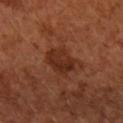Clinical impression:
This lesion was catalogued during total-body skin photography and was not selected for biopsy.
Acquisition and patient details:
This image is a 15 mm lesion crop taken from a total-body photograph. The lesion's longest dimension is about 4 mm. The lesion-visualizer software estimated an automated nevus-likeness rating near 20 out of 100 and a lesion-detection confidence of about 100/100. This is a cross-polarized tile. A female subject approximately 65 years of age. The lesion is on the right forearm.